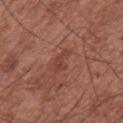The tile uses white-light illumination. The recorded lesion diameter is about 3 mm. A 15 mm close-up tile from a total-body photography series done for melanoma screening. A male patient in their mid- to late 70s. The lesion is on the chest.